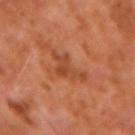Recorded during total-body skin imaging; not selected for excision or biopsy.
This is a cross-polarized tile.
A lesion tile, about 15 mm wide, cut from a 3D total-body photograph.
Measured at roughly 3.5 mm in maximum diameter.
The subject is a male approximately 60 years of age.
From the left forearm.
The total-body-photography lesion software estimated a classifier nevus-likeness of about 0/100 and a lesion-detection confidence of about 100/100.